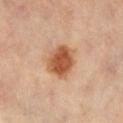The lesion was photographed on a routine skin check and not biopsied; there is no pathology result.
The recorded lesion diameter is about 4 mm.
A female patient, approximately 60 years of age.
Cropped from a total-body skin-imaging series; the visible field is about 15 mm.
From the right lower leg.
Captured under cross-polarized illumination.